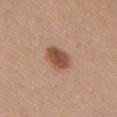Assessment:
This lesion was catalogued during total-body skin photography and was not selected for biopsy.